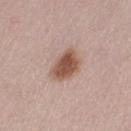Findings:
* workup: imaged on a skin check; not biopsied
* site: the left thigh
* subject: female, aged 18 to 22
* imaging modality: total-body-photography crop, ~15 mm field of view